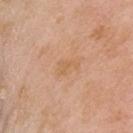| field | value |
|---|---|
| workup | imaged on a skin check; not biopsied |
| lighting | white-light |
| patient | female, aged approximately 70 |
| automated lesion analysis | a shape eccentricity near 0.8 and two-axis asymmetry of about 0.5; a color-variation rating of about 0.5/10 and a peripheral color-asymmetry measure near 0 |
| image source | ~15 mm tile from a whole-body skin photo |
| site | the head or neck |